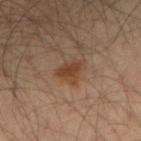subject — male, in their mid-30s; image source — ~15 mm crop, total-body skin-cancer survey; site — the right forearm.